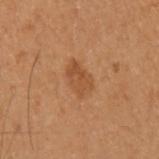Context:
Approximately 4 mm at its widest. On the left forearm. The patient is a female aged approximately 50. Cropped from a whole-body photographic skin survey; the tile spans about 15 mm. Imaged with cross-polarized lighting. Automated image analysis of the tile measured a footprint of about 7 mm² and a shape eccentricity near 0.85. The software also gave a mean CIELAB color near L≈42 a*≈20 b*≈32, a lesion–skin lightness drop of about 7, and a normalized border contrast of about 5.5.